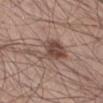Impression:
Captured during whole-body skin photography for melanoma surveillance; the lesion was not biopsied.
Image and clinical context:
From the leg. A male patient roughly 40 years of age. A lesion tile, about 15 mm wide, cut from a 3D total-body photograph.